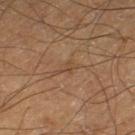A male patient aged approximately 65. The lesion is located on the left thigh. This image is a 15 mm lesion crop taken from a total-body photograph.